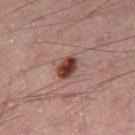<tbp_lesion>
<biopsy_status>not biopsied; imaged during a skin examination</biopsy_status>
<lesion_size>
  <long_diameter_mm_approx>3.0</long_diameter_mm_approx>
</lesion_size>
<patient>
  <sex>male</sex>
  <age_approx>50</age_approx>
</patient>
<site>leg</site>
<image>
  <source>total-body photography crop</source>
  <field_of_view_mm>15</field_of_view_mm>
</image>
<lighting>cross-polarized</lighting>
<automated_metrics>
  <nevus_likeness_0_100>100</nevus_likeness_0_100>
  <lesion_detection_confidence_0_100>100</lesion_detection_confidence_0_100>
</automated_metrics>
</tbp_lesion>